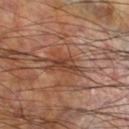The lesion was tiled from a total-body skin photograph and was not biopsied. The total-body-photography lesion software estimated lesion-presence confidence of about 75/100. A male patient, aged approximately 60. Imaged with cross-polarized lighting. On the right lower leg. A lesion tile, about 15 mm wide, cut from a 3D total-body photograph. The lesion's longest dimension is about 3 mm.